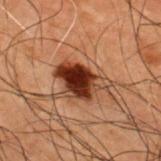notes=total-body-photography surveillance lesion; no biopsy | lesion size=~5.5 mm (longest diameter) | acquisition=total-body-photography crop, ~15 mm field of view | patient=male, in their 50s | body site=the upper back.